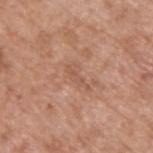subject = male, aged 63–67
site = the back
image source = total-body-photography crop, ~15 mm field of view